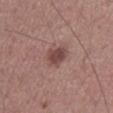Case summary:
• follow-up — catalogued during a skin exam; not biopsied
• illumination — white-light
• image — 15 mm crop, total-body photography
• subject — male, in their mid- to late 50s
• anatomic site — the back
• size — ~3 mm (longest diameter)